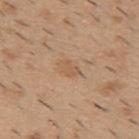lesion_size:
  long_diameter_mm_approx: 2.5
patient:
  sex: male
  age_approx: 40
image:
  source: total-body photography crop
  field_of_view_mm: 15
lighting: white-light
site: upper back